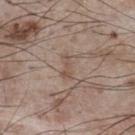Q: Was a biopsy performed?
A: catalogued during a skin exam; not biopsied
Q: Who is the patient?
A: male, in their mid-70s
Q: Where on the body is the lesion?
A: the chest
Q: Lesion size?
A: about 3 mm
Q: How was this image acquired?
A: total-body-photography crop, ~15 mm field of view